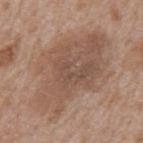Assessment:
The lesion was photographed on a routine skin check and not biopsied; there is no pathology result.
Clinical summary:
A male patient aged approximately 65. A region of skin cropped from a whole-body photographic capture, roughly 15 mm wide. The lesion is located on the back. Measured at roughly 11 mm in maximum diameter. Automated image analysis of the tile measured an outline eccentricity of about 0.8 (0 = round, 1 = elongated) and a symmetry-axis asymmetry near 0.3. And it measured a mean CIELAB color near L≈52 a*≈17 b*≈27 and about 8 CIELAB-L* units darker than the surrounding skin. It also reported a border-irregularity rating of about 4.5/10, internal color variation of about 4.5 on a 0–10 scale, and peripheral color asymmetry of about 1.5. The software also gave lesion-presence confidence of about 100/100. Imaged with white-light lighting.